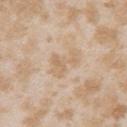- follow-up · no biopsy performed (imaged during a skin exam)
- lighting · white-light
- image · ~15 mm tile from a whole-body skin photo
- patient · female, in their mid-20s
- anatomic site · the right upper arm